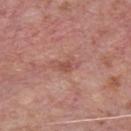Recorded during total-body skin imaging; not selected for excision or biopsy. The lesion-visualizer software estimated a lesion color around L≈52 a*≈24 b*≈27 in CIELAB, a lesion–skin lightness drop of about 8, and a normalized lesion–skin contrast near 6. The analysis additionally found border irregularity of about 5 on a 0–10 scale, a within-lesion color-variation index near 1.5/10, and a peripheral color-asymmetry measure near 0.5. Cropped from a whole-body photographic skin survey; the tile spans about 15 mm. Measured at roughly 3 mm in maximum diameter. Captured under white-light illumination. The lesion is located on the chest. A male patient in their mid-70s.